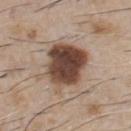Impression:
This lesion was catalogued during total-body skin photography and was not selected for biopsy.
Clinical summary:
The lesion is located on the chest. This is a white-light tile. An algorithmic analysis of the crop reported internal color variation of about 6.5 on a 0–10 scale. And it measured a classifier nevus-likeness of about 45/100 and a detector confidence of about 100 out of 100 that the crop contains a lesion. Measured at roughly 6 mm in maximum diameter. A male patient aged 58–62. Cropped from a total-body skin-imaging series; the visible field is about 15 mm.